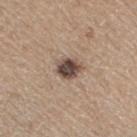Captured during whole-body skin photography for melanoma surveillance; the lesion was not biopsied. Approximately 3 mm at its widest. The patient is a female aged around 70. The tile uses white-light illumination. Cropped from a total-body skin-imaging series; the visible field is about 15 mm. An algorithmic analysis of the crop reported an area of roughly 6 mm², an eccentricity of roughly 0.45, and two-axis asymmetry of about 0.2. It also reported a color-variation rating of about 4/10 and radial color variation of about 1.5. On the right thigh.